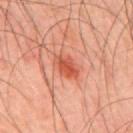- follow-up · catalogued during a skin exam; not biopsied
- patient · male, aged around 45
- anatomic site · the mid back
- acquisition · 15 mm crop, total-body photography